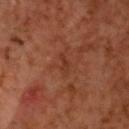Findings:
• follow-up · total-body-photography surveillance lesion; no biopsy
• patient · male, about 60 years old
• site · the head or neck
• automated metrics · an average lesion color of about L≈33 a*≈23 b*≈30 (CIELAB), about 6 CIELAB-L* units darker than the surrounding skin, and a normalized border contrast of about 5.5; a border-irregularity rating of about 4.5/10 and peripheral color asymmetry of about 0; a classifier nevus-likeness of about 0/100 and a lesion-detection confidence of about 100/100
• image source · ~15 mm crop, total-body skin-cancer survey